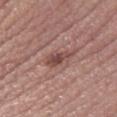No biopsy was performed on this lesion — it was imaged during a full skin examination and was not determined to be concerning.
An algorithmic analysis of the crop reported a shape-asymmetry score of about 0.35 (0 = symmetric). And it measured a border-irregularity index near 4/10, internal color variation of about 4 on a 0–10 scale, and a peripheral color-asymmetry measure near 1.
This is a white-light tile.
A female subject, about 50 years old.
About 4 mm across.
Located on the right thigh.
A roughly 15 mm field-of-view crop from a total-body skin photograph.